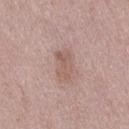<lesion>
<biopsy_status>not biopsied; imaged during a skin examination</biopsy_status>
<site>right thigh</site>
<automated_metrics>
  <nevus_likeness_0_100>5</nevus_likeness_0_100>
</automated_metrics>
<image>
  <source>total-body photography crop</source>
  <field_of_view_mm>15</field_of_view_mm>
</image>
<lesion_size>
  <long_diameter_mm_approx>3.5</long_diameter_mm_approx>
</lesion_size>
<lighting>white-light</lighting>
<patient>
  <sex>female</sex>
  <age_approx>40</age_approx>
</patient>
</lesion>